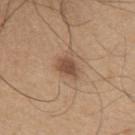Recorded during total-body skin imaging; not selected for excision or biopsy.
The recorded lesion diameter is about 3 mm.
The lesion is located on the chest.
A male subject, in their mid-60s.
A close-up tile cropped from a whole-body skin photograph, about 15 mm across.